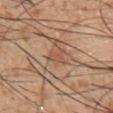Q: Was a biopsy performed?
A: imaged on a skin check; not biopsied
Q: What is the imaging modality?
A: ~15 mm crop, total-body skin-cancer survey
Q: What lighting was used for the tile?
A: white-light illumination
Q: What is the anatomic site?
A: the chest
Q: What did automated image analysis measure?
A: a footprint of about 3 mm², an outline eccentricity of about 0.9 (0 = round, 1 = elongated), and a shape-asymmetry score of about 0.55 (0 = symmetric); border irregularity of about 6 on a 0–10 scale, internal color variation of about 1.5 on a 0–10 scale, and radial color variation of about 0.5; a detector confidence of about 55 out of 100 that the crop contains a lesion
Q: Who is the patient?
A: male, aged approximately 45